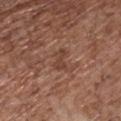<case>
<biopsy_status>not biopsied; imaged during a skin examination</biopsy_status>
<site>front of the torso</site>
<lesion_size>
  <long_diameter_mm_approx>2.5</long_diameter_mm_approx>
</lesion_size>
<lighting>white-light</lighting>
<image>
  <source>total-body photography crop</source>
  <field_of_view_mm>15</field_of_view_mm>
</image>
<patient>
  <sex>male</sex>
  <age_approx>70</age_approx>
</patient>
</case>